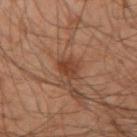Assessment: Part of a total-body skin-imaging series; this lesion was reviewed on a skin check and was not flagged for biopsy. Clinical summary: A 15 mm close-up tile from a total-body photography series done for melanoma screening. A male subject approximately 50 years of age. The lesion is on the left arm.